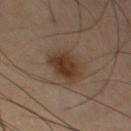follow-up: imaged on a skin check; not biopsied | TBP lesion metrics: a lesion area of about 11 mm², an eccentricity of roughly 0.7, and a shape-asymmetry score of about 0.2 (0 = symmetric); a mean CIELAB color near L≈35 a*≈16 b*≈26 and a lesion–skin lightness drop of about 10 | subject: male, approximately 65 years of age | lesion size: ~4.5 mm (longest diameter) | image source: ~15 mm tile from a whole-body skin photo | illumination: cross-polarized | body site: the left thigh.